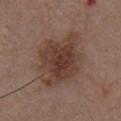biopsy_status: not biopsied; imaged during a skin examination
site: chest
patient:
  sex: male
  age_approx: 55
image:
  source: total-body photography crop
  field_of_view_mm: 15
lesion_size:
  long_diameter_mm_approx: 8.0
lighting: white-light